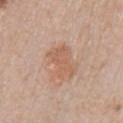follow-up: imaged on a skin check; not biopsied
size: about 4 mm
image source: total-body-photography crop, ~15 mm field of view
subject: female, approximately 65 years of age
illumination: white-light
location: the arm